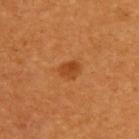biopsy status = catalogued during a skin exam; not biopsied
acquisition = total-body-photography crop, ~15 mm field of view
patient = male, aged 58–62
illumination = cross-polarized illumination
site = the upper back